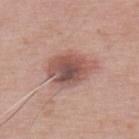The lesion was tiled from a total-body skin photograph and was not biopsied. A male patient, aged 63–67. The tile uses white-light illumination. Cropped from a total-body skin-imaging series; the visible field is about 15 mm. On the upper back.